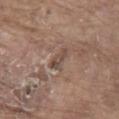Recorded during total-body skin imaging; not selected for excision or biopsy. The tile uses white-light illumination. The lesion is on the abdomen. The total-body-photography lesion software estimated an area of roughly 3 mm², an eccentricity of roughly 0.95, and a shape-asymmetry score of about 0.5 (0 = symmetric). It also reported roughly 8 lightness units darker than nearby skin and a normalized lesion–skin contrast near 6.5. The software also gave a border-irregularity rating of about 5.5/10, a color-variation rating of about 0/10, and peripheral color asymmetry of about 0. It also reported a nevus-likeness score of about 0/100 and a lesion-detection confidence of about 70/100. A male patient, approximately 80 years of age. A lesion tile, about 15 mm wide, cut from a 3D total-body photograph. About 3 mm across.